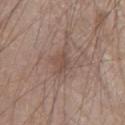This lesion was catalogued during total-body skin photography and was not selected for biopsy.
The total-body-photography lesion software estimated a lesion area of about 3.5 mm², a shape eccentricity near 0.8, and a shape-asymmetry score of about 0.4 (0 = symmetric). The analysis additionally found an average lesion color of about L≈48 a*≈16 b*≈24 (CIELAB), a lesion–skin lightness drop of about 7, and a normalized lesion–skin contrast near 5.5.
A region of skin cropped from a whole-body photographic capture, roughly 15 mm wide.
On the front of the torso.
A male subject aged approximately 80.